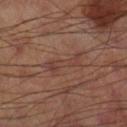<record>
<biopsy_status>not biopsied; imaged during a skin examination</biopsy_status>
<lighting>cross-polarized</lighting>
<lesion_size>
  <long_diameter_mm_approx>5.0</long_diameter_mm_approx>
</lesion_size>
<image>
  <source>total-body photography crop</source>
  <field_of_view_mm>15</field_of_view_mm>
</image>
<site>leg</site>
<automated_metrics>
  <vs_skin_darker_L>6.0</vs_skin_darker_L>
  <vs_skin_contrast_norm>5.5</vs_skin_contrast_norm>
  <border_irregularity_0_10>6.5</border_irregularity_0_10>
  <color_variation_0_10>1.5</color_variation_0_10>
  <peripheral_color_asymmetry>0.5</peripheral_color_asymmetry>
  <nevus_likeness_0_100>0</nevus_likeness_0_100>
  <lesion_detection_confidence_0_100>95</lesion_detection_confidence_0_100>
</automated_metrics>
</record>